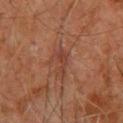Impression: No biopsy was performed on this lesion — it was imaged during a full skin examination and was not determined to be concerning. Clinical summary: A male patient aged approximately 60. Measured at roughly 3 mm in maximum diameter. The lesion is on the upper back. The lesion-visualizer software estimated a lesion area of about 3.5 mm². The analysis additionally found a lesion color around L≈36 a*≈22 b*≈26 in CIELAB, roughly 7 lightness units darker than nearby skin, and a lesion-to-skin contrast of about 6 (normalized; higher = more distinct). And it measured a border-irregularity rating of about 5.5/10 and a within-lesion color-variation index near 2/10. The analysis additionally found a classifier nevus-likeness of about 0/100. Cropped from a whole-body photographic skin survey; the tile spans about 15 mm.